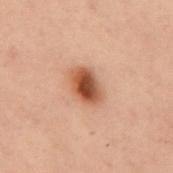biopsy status: imaged on a skin check; not biopsied | tile lighting: cross-polarized illumination | site: the back | patient: female, in their mid- to late 50s | image source: ~15 mm tile from a whole-body skin photo | TBP lesion metrics: a border-irregularity rating of about 1.5/10; a classifier nevus-likeness of about 100/100 and lesion-presence confidence of about 100/100 | diameter: ~4 mm (longest diameter).